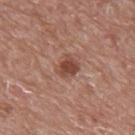Findings:
* workup — catalogued during a skin exam; not biopsied
* tile lighting — white-light illumination
* diameter — ~2.5 mm (longest diameter)
* image source — ~15 mm crop, total-body skin-cancer survey
* anatomic site — the upper back
* subject — male, aged 68 to 72